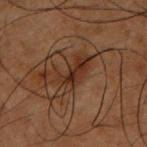  biopsy_status: not biopsied; imaged during a skin examination
  image:
    source: total-body photography crop
    field_of_view_mm: 15
  patient:
    sex: male
    age_approx: 50
  site: upper back